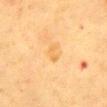biopsy_status: not biopsied; imaged during a skin examination
lighting: cross-polarized
patient:
  sex: female
  age_approx: 55
site: chest
image:
  source: total-body photography crop
  field_of_view_mm: 15
automated_metrics:
  cielab_L: 59
  cielab_a: 17
  cielab_b: 42
  vs_skin_contrast_norm: 5.5
lesion_size:
  long_diameter_mm_approx: 2.5